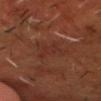The lesion was photographed on a routine skin check and not biopsied; there is no pathology result. On the head or neck. The recorded lesion diameter is about 4 mm. A male subject, in their 50s. The total-body-photography lesion software estimated lesion-presence confidence of about 95/100. A 15 mm close-up tile from a total-body photography series done for melanoma screening. Captured under cross-polarized illumination.